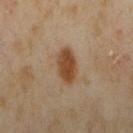No biopsy was performed on this lesion — it was imaged during a full skin examination and was not determined to be concerning.